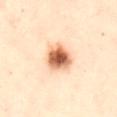Imaged during a routine full-body skin examination; the lesion was not biopsied and no histopathology is available.
A 15 mm crop from a total-body photograph taken for skin-cancer surveillance.
On the front of the torso.
The lesion-visualizer software estimated border irregularity of about 2 on a 0–10 scale, a within-lesion color-variation index near 6/10, and peripheral color asymmetry of about 1.5. It also reported a nevus-likeness score of about 100/100.
A female subject aged around 30.
Measured at roughly 4 mm in maximum diameter.